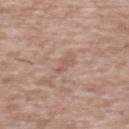Notes:
– notes — no biopsy performed (imaged during a skin exam)
– subject — male, roughly 45 years of age
– body site — the mid back
– lesion diameter — ~2.5 mm (longest diameter)
– image — ~15 mm crop, total-body skin-cancer survey